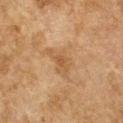<lesion>
<biopsy_status>not biopsied; imaged during a skin examination</biopsy_status>
<image>
  <source>total-body photography crop</source>
  <field_of_view_mm>15</field_of_view_mm>
</image>
<patient>
  <sex>female</sex>
  <age_approx>60</age_approx>
</patient>
<site>left forearm</site>
</lesion>